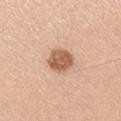<tbp_lesion>
<biopsy_status>not biopsied; imaged during a skin examination</biopsy_status>
<image>
  <source>total-body photography crop</source>
  <field_of_view_mm>15</field_of_view_mm>
</image>
<lesion_size>
  <long_diameter_mm_approx>3.5</long_diameter_mm_approx>
</lesion_size>
<automated_metrics>
  <area_mm2_approx>8.0</area_mm2_approx>
  <eccentricity>0.5</eccentricity>
  <shape_asymmetry>0.1</shape_asymmetry>
</automated_metrics>
<patient>
  <sex>male</sex>
  <age_approx>40</age_approx>
</patient>
<site>right upper arm</site>
<lighting>white-light</lighting>
</tbp_lesion>